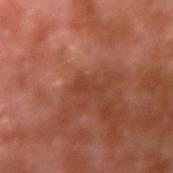workup — imaged on a skin check; not biopsied.